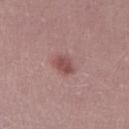workup: no biopsy performed (imaged during a skin exam)
tile lighting: white-light
image: total-body-photography crop, ~15 mm field of view
subject: male, roughly 30 years of age
diameter: ≈3 mm